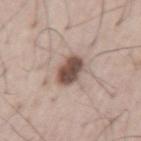biopsy status: total-body-photography surveillance lesion; no biopsy
lesion size: ~3.5 mm (longest diameter)
image-analysis metrics: an area of roughly 8 mm² and two-axis asymmetry of about 0.15; a mean CIELAB color near L≈50 a*≈17 b*≈23, about 17 CIELAB-L* units darker than the surrounding skin, and a lesion-to-skin contrast of about 11.5 (normalized; higher = more distinct); border irregularity of about 1.5 on a 0–10 scale, a within-lesion color-variation index near 5/10, and peripheral color asymmetry of about 1.5
image source: 15 mm crop, total-body photography
tile lighting: white-light
patient: male, in their mid-50s